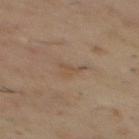<lesion>
<biopsy_status>not biopsied; imaged during a skin examination</biopsy_status>
<image>
  <source>total-body photography crop</source>
  <field_of_view_mm>15</field_of_view_mm>
</image>
<site>mid back</site>
<lighting>cross-polarized</lighting>
<patient>
  <sex>male</sex>
  <age_approx>55</age_approx>
</patient>
<automated_metrics>
  <area_mm2_approx>3.5</area_mm2_approx>
  <eccentricity>0.75</eccentricity>
  <shape_asymmetry>0.55</shape_asymmetry>
  <cielab_L>47</cielab_L>
  <cielab_a>14</cielab_a>
  <cielab_b>28</cielab_b>
  <vs_skin_darker_L>5.0</vs_skin_darker_L>
  <vs_skin_contrast_norm>4.5</vs_skin_contrast_norm>
  <border_irregularity_0_10>5.5</border_irregularity_0_10>
  <color_variation_0_10>1.0</color_variation_0_10>
  <peripheral_color_asymmetry>0.5</peripheral_color_asymmetry>
  <nevus_likeness_0_100>0</nevus_likeness_0_100>
  <lesion_detection_confidence_0_100>100</lesion_detection_confidence_0_100>
</automated_metrics>
<lesion_size>
  <long_diameter_mm_approx>3.0</long_diameter_mm_approx>
</lesion_size>
</lesion>